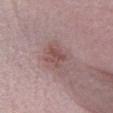Findings:
- workup — catalogued during a skin exam; not biopsied
- site — the leg
- subject — male, roughly 50 years of age
- automated lesion analysis — an area of roughly 4.5 mm², an eccentricity of roughly 0.5, and a symmetry-axis asymmetry near 0.4; an automated nevus-likeness rating near 10 out of 100
- acquisition — ~15 mm tile from a whole-body skin photo